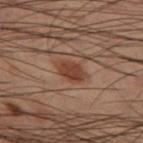Image and clinical context:
Cropped from a total-body skin-imaging series; the visible field is about 15 mm. A male patient, aged 53–57. On the left thigh. Automated image analysis of the tile measured a lesion area of about 5.5 mm², a shape eccentricity near 0.7, and a symmetry-axis asymmetry near 0.15. And it measured a border-irregularity index near 1.5/10, internal color variation of about 2 on a 0–10 scale, and peripheral color asymmetry of about 0.5. The software also gave a nevus-likeness score of about 85/100. Imaged with cross-polarized lighting.